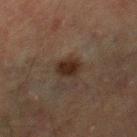Impression: No biopsy was performed on this lesion — it was imaged during a full skin examination and was not determined to be concerning. Image and clinical context: A male patient approximately 65 years of age. From the leg. Cropped from a whole-body photographic skin survey; the tile spans about 15 mm.